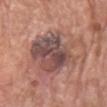Q: Where on the body is the lesion?
A: the chest
Q: What kind of image is this?
A: ~15 mm crop, total-body skin-cancer survey
Q: What did automated image analysis measure?
A: an area of roughly 32 mm², an eccentricity of roughly 0.5, and two-axis asymmetry of about 0.25; an average lesion color of about L≈49 a*≈20 b*≈22 (CIELAB), about 11 CIELAB-L* units darker than the surrounding skin, and a normalized lesion–skin contrast near 8.5; a color-variation rating of about 8/10 and radial color variation of about 2.5; a detector confidence of about 95 out of 100 that the crop contains a lesion
Q: Illumination type?
A: white-light illumination
Q: Patient demographics?
A: male, aged 78–82